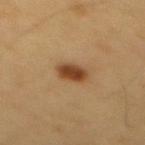biopsy status: total-body-photography surveillance lesion; no biopsy
tile lighting: cross-polarized
subject: male, approximately 60 years of age
anatomic site: the mid back
automated metrics: a footprint of about 6 mm², an eccentricity of roughly 0.75, and a shape-asymmetry score of about 0.15 (0 = symmetric); a mean CIELAB color near L≈44 a*≈20 b*≈35, a lesion–skin lightness drop of about 14, and a lesion-to-skin contrast of about 10.5 (normalized; higher = more distinct); a border-irregularity rating of about 2/10 and a color-variation rating of about 3.5/10
lesion size: ≈3.5 mm
image source: ~15 mm crop, total-body skin-cancer survey